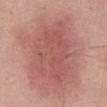{"biopsy_status": "not biopsied; imaged during a skin examination", "image": {"source": "total-body photography crop", "field_of_view_mm": 15}, "lighting": "white-light", "lesion_size": {"long_diameter_mm_approx": 8.5}, "patient": {"sex": "male", "age_approx": 55}, "automated_metrics": {"area_mm2_approx": 31.0, "border_irregularity_0_10": 3.5, "color_variation_0_10": 3.0, "peripheral_color_asymmetry": 1.0}, "site": "abdomen"}